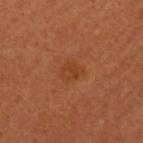Imaged during a routine full-body skin examination; the lesion was not biopsied and no histopathology is available.
Captured under cross-polarized illumination.
Measured at roughly 2.5 mm in maximum diameter.
The patient is a female in their 50s.
Automated image analysis of the tile measured a border-irregularity rating of about 2.5/10, internal color variation of about 2.5 on a 0–10 scale, and radial color variation of about 1. And it measured a nevus-likeness score of about 5/100 and a detector confidence of about 100 out of 100 that the crop contains a lesion.
Cropped from a whole-body photographic skin survey; the tile spans about 15 mm.